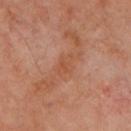Clinical impression:
Captured during whole-body skin photography for melanoma surveillance; the lesion was not biopsied.
Context:
A 15 mm crop from a total-body photograph taken for skin-cancer surveillance. A male subject in their mid-60s. Located on the chest. Longest diameter approximately 3 mm.